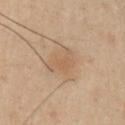follow-up = total-body-photography surveillance lesion; no biopsy | anatomic site = the left upper arm | image source = 15 mm crop, total-body photography | subject = male, about 40 years old.